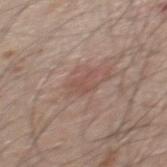This lesion was catalogued during total-body skin photography and was not selected for biopsy. Imaged with white-light lighting. The total-body-photography lesion software estimated a footprint of about 5 mm², an outline eccentricity of about 0.75 (0 = round, 1 = elongated), and a shape-asymmetry score of about 0.35 (0 = symmetric). It also reported border irregularity of about 4.5 on a 0–10 scale and a color-variation rating of about 1.5/10. Cropped from a total-body skin-imaging series; the visible field is about 15 mm. On the back. The lesion's longest dimension is about 3.5 mm. A male patient, aged approximately 50.